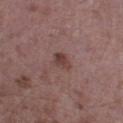Recorded during total-body skin imaging; not selected for excision or biopsy.
A close-up tile cropped from a whole-body skin photograph, about 15 mm across.
The lesion is on the left lower leg.
The tile uses white-light illumination.
The lesion-visualizer software estimated a normalized lesion–skin contrast near 7.5. The software also gave a border-irregularity index near 2.5/10. The software also gave a classifier nevus-likeness of about 15/100.
A male patient aged around 50.